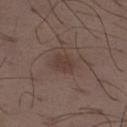Findings:
– follow-up: catalogued during a skin exam; not biopsied